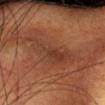| feature | finding |
|---|---|
| acquisition | total-body-photography crop, ~15 mm field of view |
| anatomic site | the head or neck |
| subject | male, aged around 55 |